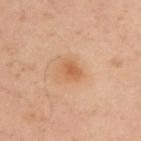The lesion was photographed on a routine skin check and not biopsied; there is no pathology result.
Cropped from a whole-body photographic skin survey; the tile spans about 15 mm.
Captured under cross-polarized illumination.
The lesion is located on the upper back.
A male patient, about 45 years old.